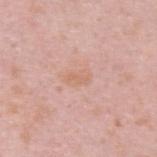Background:
The lesion is located on the upper back. This is a white-light tile. A male subject, in their mid-30s. The lesion-visualizer software estimated an area of roughly 3.5 mm², an eccentricity of roughly 0.85, and two-axis asymmetry of about 0.2. And it measured an average lesion color of about L≈65 a*≈21 b*≈30 (CIELAB) and a lesion–skin lightness drop of about 5. A close-up tile cropped from a whole-body skin photograph, about 15 mm across. The lesion's longest dimension is about 2.5 mm.